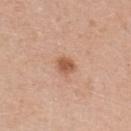Q: Was this lesion biopsied?
A: catalogued during a skin exam; not biopsied
Q: Who is the patient?
A: female, aged 38 to 42
Q: What is the imaging modality?
A: ~15 mm tile from a whole-body skin photo
Q: What is the lesion's diameter?
A: ≈2.5 mm
Q: Where on the body is the lesion?
A: the upper back